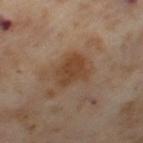biopsy status: no biopsy performed (imaged during a skin exam); image: total-body-photography crop, ~15 mm field of view; lighting: cross-polarized; subject: female, in their mid-50s; lesion size: about 3.5 mm; site: the leg.